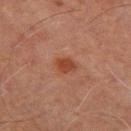Impression: The lesion was tiled from a total-body skin photograph and was not biopsied. Clinical summary: The lesion-visualizer software estimated a lesion area of about 3.5 mm², a shape eccentricity near 0.7, and a shape-asymmetry score of about 0.25 (0 = symmetric). And it measured a mean CIELAB color near L≈35 a*≈23 b*≈28 and a lesion–skin lightness drop of about 8. On the right thigh. The subject is a male in their 70s. A lesion tile, about 15 mm wide, cut from a 3D total-body photograph. Measured at roughly 2.5 mm in maximum diameter. This is a cross-polarized tile.